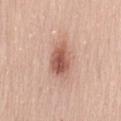{"biopsy_status": "not biopsied; imaged during a skin examination", "lighting": "white-light", "lesion_size": {"long_diameter_mm_approx": 4.0}, "image": {"source": "total-body photography crop", "field_of_view_mm": 15}, "patient": {"sex": "female", "age_approx": 45}, "site": "back"}